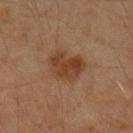Part of a total-body skin-imaging series; this lesion was reviewed on a skin check and was not flagged for biopsy. A male subject, about 60 years old. An algorithmic analysis of the crop reported a footprint of about 11 mm² and a shape-asymmetry score of about 0.3 (0 = symmetric). The analysis additionally found roughly 8 lightness units darker than nearby skin and a lesion-to-skin contrast of about 8 (normalized; higher = more distinct). Approximately 4 mm at its widest. Cropped from a total-body skin-imaging series; the visible field is about 15 mm. Located on the left upper arm. Captured under cross-polarized illumination.